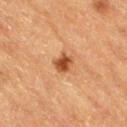- follow-up · imaged on a skin check; not biopsied
- automated lesion analysis · an area of roughly 4.5 mm², an eccentricity of roughly 0.7, and a symmetry-axis asymmetry near 0.3; an average lesion color of about L≈45 a*≈23 b*≈36 (CIELAB), a lesion–skin lightness drop of about 13, and a lesion-to-skin contrast of about 9.5 (normalized; higher = more distinct); a border-irregularity rating of about 2.5/10 and radial color variation of about 1
- patient · male, aged 83 or older
- lesion diameter · ≈2.5 mm
- acquisition · ~15 mm crop, total-body skin-cancer survey
- body site · the lower back
- lighting · cross-polarized illumination